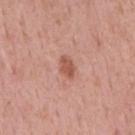Assessment:
The lesion was photographed on a routine skin check and not biopsied; there is no pathology result.
Context:
Measured at roughly 2.5 mm in maximum diameter. The lesion is located on the mid back. This image is a 15 mm lesion crop taken from a total-body photograph. The tile uses white-light illumination. A male patient about 55 years old.